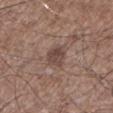| key | value |
|---|---|
| biopsy status | catalogued during a skin exam; not biopsied |
| lesion diameter | about 3 mm |
| lighting | white-light illumination |
| anatomic site | the left lower leg |
| acquisition | 15 mm crop, total-body photography |
| subject | male, about 60 years old |